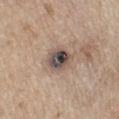Notes:
– workup: total-body-photography surveillance lesion; no biopsy
– size: about 3.5 mm
– acquisition: 15 mm crop, total-body photography
– anatomic site: the left lower leg
– subject: male, aged 68–72